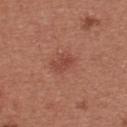biopsy_status: not biopsied; imaged during a skin examination
image:
  source: total-body photography crop
  field_of_view_mm: 15
patient:
  sex: female
  age_approx: 25
site: upper back